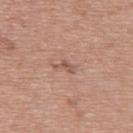{
  "biopsy_status": "not biopsied; imaged during a skin examination",
  "patient": {
    "sex": "female",
    "age_approx": 60
  },
  "automated_metrics": {
    "cielab_L": 54,
    "cielab_a": 21,
    "cielab_b": 27,
    "vs_skin_darker_L": 9.0,
    "vs_skin_contrast_norm": 6.0,
    "border_irregularity_0_10": 5.5,
    "color_variation_0_10": 0.0,
    "nevus_likeness_0_100": 0
  },
  "image": {
    "source": "total-body photography crop",
    "field_of_view_mm": 15
  },
  "lesion_size": {
    "long_diameter_mm_approx": 2.5
  },
  "site": "upper back"
}